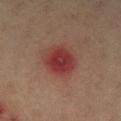The lesion was photographed on a routine skin check and not biopsied; there is no pathology result.
Measured at roughly 3.5 mm in maximum diameter.
Located on the left lower leg.
A patient approximately 60 years of age.
Automated image analysis of the tile measured border irregularity of about 1.5 on a 0–10 scale and a within-lesion color-variation index near 4/10. The software also gave a classifier nevus-likeness of about 0/100 and a detector confidence of about 100 out of 100 that the crop contains a lesion.
A close-up tile cropped from a whole-body skin photograph, about 15 mm across.
The tile uses cross-polarized illumination.